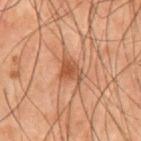Q: Was this lesion biopsied?
A: imaged on a skin check; not biopsied
Q: What did automated image analysis measure?
A: a lesion area of about 5.5 mm², an outline eccentricity of about 0.65 (0 = round, 1 = elongated), and two-axis asymmetry of about 0.4; a lesion color around L≈40 a*≈20 b*≈28 in CIELAB, roughly 8 lightness units darker than nearby skin, and a lesion-to-skin contrast of about 7 (normalized; higher = more distinct)
Q: What kind of image is this?
A: 15 mm crop, total-body photography
Q: Patient demographics?
A: male, aged 68 to 72
Q: How was the tile lit?
A: cross-polarized illumination
Q: How large is the lesion?
A: ≈3 mm
Q: Where on the body is the lesion?
A: the front of the torso